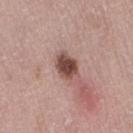Assessment: No biopsy was performed on this lesion — it was imaged during a full skin examination and was not determined to be concerning. Acquisition and patient details: The lesion is located on the right thigh. This is a white-light tile. This image is a 15 mm lesion crop taken from a total-body photograph. A female subject, aged around 50. Approximately 3.5 mm at its widest.